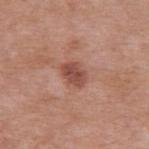Imaged during a routine full-body skin examination; the lesion was not biopsied and no histopathology is available.
An algorithmic analysis of the crop reported a lesion area of about 5.5 mm² and a symmetry-axis asymmetry near 0.25. It also reported border irregularity of about 2 on a 0–10 scale. It also reported an automated nevus-likeness rating near 5 out of 100 and lesion-presence confidence of about 100/100.
This is a white-light tile.
The patient is a male in their mid-50s.
A region of skin cropped from a whole-body photographic capture, roughly 15 mm wide.
The lesion is located on the left upper arm.